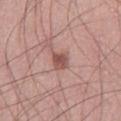Captured under white-light illumination. The lesion is located on the leg. Longest diameter approximately 3 mm. A 15 mm close-up extracted from a 3D total-body photography capture. Automated image analysis of the tile measured a border-irregularity rating of about 2.5/10, a color-variation rating of about 3/10, and a peripheral color-asymmetry measure near 1. A male patient, about 60 years old.